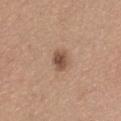{
  "biopsy_status": "not biopsied; imaged during a skin examination",
  "site": "back",
  "image": {
    "source": "total-body photography crop",
    "field_of_view_mm": 15
  },
  "patient": {
    "sex": "female",
    "age_approx": 35
  },
  "lesion_size": {
    "long_diameter_mm_approx": 2.5
  },
  "lighting": "white-light"
}